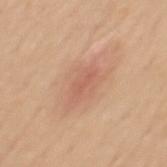biopsy status: no biopsy performed (imaged during a skin exam) | tile lighting: white-light illumination | site: the back | image-analysis metrics: a lesion color around L≈59 a*≈24 b*≈31 in CIELAB; a border-irregularity rating of about 4/10, a color-variation rating of about 0.5/10, and peripheral color asymmetry of about 0 | acquisition: 15 mm crop, total-body photography | subject: male, aged 48 to 52.